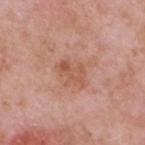Q: Is there a histopathology result?
A: imaged on a skin check; not biopsied
Q: Patient demographics?
A: male, aged around 50
Q: Lesion location?
A: the chest
Q: Automated lesion metrics?
A: a lesion area of about 6.5 mm², an outline eccentricity of about 0.8 (0 = round, 1 = elongated), and two-axis asymmetry of about 0.4; an automated nevus-likeness rating near 0 out of 100 and a lesion-detection confidence of about 100/100
Q: What lighting was used for the tile?
A: white-light
Q: What is the imaging modality?
A: ~15 mm tile from a whole-body skin photo
Q: How large is the lesion?
A: ≈3.5 mm